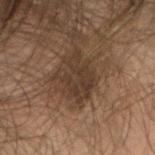biopsy status = total-body-photography surveillance lesion; no biopsy | patient = female, approximately 20 years of age | imaging modality = ~15 mm tile from a whole-body skin photo | size = ≈5 mm | location = the head or neck | lighting = cross-polarized.